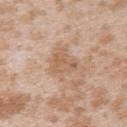workup: catalogued during a skin exam; not biopsied
site: the upper back
subject: female, about 25 years old
image-analysis metrics: a footprint of about 7 mm², an eccentricity of roughly 0.5, and a shape-asymmetry score of about 0.4 (0 = symmetric); a lesion color around L≈60 a*≈18 b*≈31 in CIELAB and a normalized border contrast of about 5.5; a border-irregularity index near 4.5/10 and radial color variation of about 1; a classifier nevus-likeness of about 0/100 and a lesion-detection confidence of about 100/100
image: total-body-photography crop, ~15 mm field of view
tile lighting: white-light
lesion diameter: about 3.5 mm